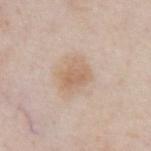Imaged with white-light lighting. Measured at roughly 3.5 mm in maximum diameter. The patient is a male about 55 years old. An algorithmic analysis of the crop reported an area of roughly 8 mm² and two-axis asymmetry of about 0.15. It also reported an average lesion color of about L≈64 a*≈16 b*≈30 (CIELAB). The analysis additionally found a classifier nevus-likeness of about 15/100. Located on the chest. A region of skin cropped from a whole-body photographic capture, roughly 15 mm wide.